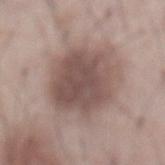<case>
<biopsy_status>not biopsied; imaged during a skin examination</biopsy_status>
<lesion_size>
  <long_diameter_mm_approx>7.0</long_diameter_mm_approx>
</lesion_size>
<site>abdomen</site>
<patient>
  <sex>male</sex>
  <age_approx>55</age_approx>
</patient>
<image>
  <source>total-body photography crop</source>
  <field_of_view_mm>15</field_of_view_mm>
</image>
</case>